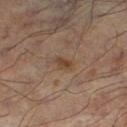The lesion was photographed on a routine skin check and not biopsied; there is no pathology result. A 15 mm crop from a total-body photograph taken for skin-cancer surveillance. The lesion is located on the leg. A male subject, approximately 55 years of age.